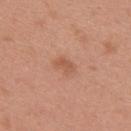Q: Was this lesion biopsied?
A: no biopsy performed (imaged during a skin exam)
Q: Patient demographics?
A: female, aged 38–42
Q: Where on the body is the lesion?
A: the back
Q: How was this image acquired?
A: total-body-photography crop, ~15 mm field of view